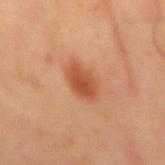Assessment: No biopsy was performed on this lesion — it was imaged during a full skin examination and was not determined to be concerning. Clinical summary: About 4.5 mm across. Imaged with cross-polarized lighting. A 15 mm close-up extracted from a 3D total-body photography capture. The subject is a male aged 63 to 67. Automated image analysis of the tile measured an area of roughly 8.5 mm², an eccentricity of roughly 0.85, and a symmetry-axis asymmetry near 0.25. The analysis additionally found a lesion color around L≈53 a*≈28 b*≈37 in CIELAB, about 11 CIELAB-L* units darker than the surrounding skin, and a normalized lesion–skin contrast near 8. The lesion is on the back.